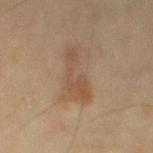{"biopsy_status": "not biopsied; imaged during a skin examination", "image": {"source": "total-body photography crop", "field_of_view_mm": 15}, "site": "left thigh", "patient": {"sex": "female", "age_approx": 55}}